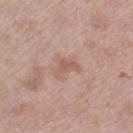The lesion was tiled from a total-body skin photograph and was not biopsied. Located on the right lower leg. The tile uses white-light illumination. Automated tile analysis of the lesion measured a footprint of about 4 mm², an eccentricity of roughly 0.75, and two-axis asymmetry of about 0.5. The software also gave a mean CIELAB color near L≈57 a*≈20 b*≈26, about 8 CIELAB-L* units darker than the surrounding skin, and a normalized lesion–skin contrast near 5.5. The analysis additionally found border irregularity of about 4.5 on a 0–10 scale, internal color variation of about 1 on a 0–10 scale, and radial color variation of about 0.5. The analysis additionally found a lesion-detection confidence of about 100/100. A female patient, in their 50s. A close-up tile cropped from a whole-body skin photograph, about 15 mm across. About 3 mm across.